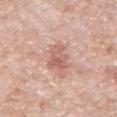Part of a total-body skin-imaging series; this lesion was reviewed on a skin check and was not flagged for biopsy.
The subject is a male about 65 years old.
The lesion is located on the abdomen.
Cropped from a total-body skin-imaging series; the visible field is about 15 mm.
This is a white-light tile.
Automated image analysis of the tile measured a lesion color around L≈61 a*≈23 b*≈26 in CIELAB.